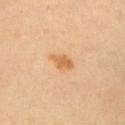Q: Was a biopsy performed?
A: total-body-photography surveillance lesion; no biopsy